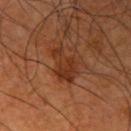Part of a total-body skin-imaging series; this lesion was reviewed on a skin check and was not flagged for biopsy. A male patient in their mid-60s. The recorded lesion diameter is about 4.5 mm. The lesion is on the right upper arm. A 15 mm crop from a total-body photograph taken for skin-cancer surveillance. The tile uses cross-polarized illumination.